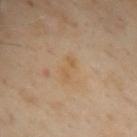{"biopsy_status": "not biopsied; imaged during a skin examination", "image": {"source": "total-body photography crop", "field_of_view_mm": 15}, "site": "upper back", "patient": {"sex": "female", "age_approx": 60}, "lighting": "cross-polarized", "automated_metrics": {"border_irregularity_0_10": 4.5, "color_variation_0_10": 0.0, "peripheral_color_asymmetry": 0.0, "nevus_likeness_0_100": 0, "lesion_detection_confidence_0_100": 100}, "lesion_size": {"long_diameter_mm_approx": 3.0}}